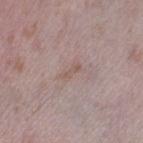Assessment: Imaged during a routine full-body skin examination; the lesion was not biopsied and no histopathology is available. Background: Longest diameter approximately 3 mm. A female subject, approximately 40 years of age. On the left thigh. A roughly 15 mm field-of-view crop from a total-body skin photograph. This is a white-light tile.